From the right upper arm · the subject is a male roughly 60 years of age · a roughly 15 mm field-of-view crop from a total-body skin photograph: 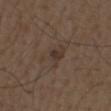lesion size: ≈2 mm
tile lighting: white-light
automated metrics: a footprint of about 2 mm², an eccentricity of roughly 0.8, and a shape-asymmetry score of about 0.25 (0 = symmetric); an average lesion color of about L≈32 a*≈13 b*≈21 (CIELAB), roughly 7 lightness units darker than nearby skin, and a lesion-to-skin contrast of about 7.5 (normalized; higher = more distinct); border irregularity of about 2 on a 0–10 scale, a within-lesion color-variation index near 0/10, and peripheral color asymmetry of about 0
pathology: a nodular basal cell carcinoma